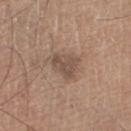Recorded during total-body skin imaging; not selected for excision or biopsy.
A male subject, aged around 65.
A 15 mm crop from a total-body photograph taken for skin-cancer surveillance.
On the right lower leg.